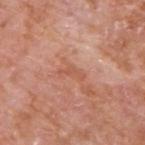Recorded during total-body skin imaging; not selected for excision or biopsy. A male subject, in their mid- to late 60s. Measured at roughly 3 mm in maximum diameter. From the upper back. A 15 mm close-up extracted from a 3D total-body photography capture. The tile uses white-light illumination.